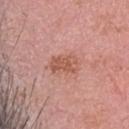{"image": {"source": "total-body photography crop", "field_of_view_mm": 15}, "patient": {"sex": "female", "age_approx": 40}, "site": "head or neck"}